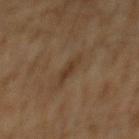{
  "biopsy_status": "not biopsied; imaged during a skin examination",
  "site": "mid back",
  "image": {
    "source": "total-body photography crop",
    "field_of_view_mm": 15
  },
  "patient": {
    "sex": "male",
    "age_approx": 60
  }
}